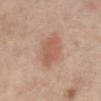Impression: Captured during whole-body skin photography for melanoma surveillance; the lesion was not biopsied. Background: Captured under white-light illumination. From the abdomen. A female subject, approximately 65 years of age. The total-body-photography lesion software estimated an outline eccentricity of about 0.8 (0 = round, 1 = elongated) and two-axis asymmetry of about 0.2. It also reported a mean CIELAB color near L≈57 a*≈22 b*≈29, about 9 CIELAB-L* units darker than the surrounding skin, and a normalized border contrast of about 6. It also reported a color-variation rating of about 2.5/10 and peripheral color asymmetry of about 1. Cropped from a whole-body photographic skin survey; the tile spans about 15 mm.